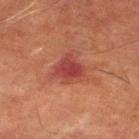Notes:
– notes · total-body-photography surveillance lesion; no biopsy
– body site · the leg
– lighting · cross-polarized illumination
– automated metrics · a shape eccentricity near 0.15 and two-axis asymmetry of about 0.35; a lesion color around L≈36 a*≈28 b*≈24 in CIELAB and about 8 CIELAB-L* units darker than the surrounding skin
– image source · total-body-photography crop, ~15 mm field of view
– subject · male, aged 68–72
– size · about 3.5 mm